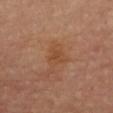Recorded during total-body skin imaging; not selected for excision or biopsy. Imaged with cross-polarized lighting. A female subject, in their mid- to late 60s. The total-body-photography lesion software estimated a footprint of about 4.5 mm², an eccentricity of roughly 0.65, and a shape-asymmetry score of about 0.35 (0 = symmetric). About 3 mm across. A 15 mm crop from a total-body photograph taken for skin-cancer surveillance. On the right forearm.